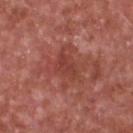Impression:
This lesion was catalogued during total-body skin photography and was not selected for biopsy.
Clinical summary:
An algorithmic analysis of the crop reported an average lesion color of about L≈42 a*≈29 b*≈27 (CIELAB). A male subject aged approximately 65. Cropped from a total-body skin-imaging series; the visible field is about 15 mm. Captured under white-light illumination. On the chest. The recorded lesion diameter is about 4 mm.